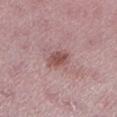Q: Was this lesion biopsied?
A: catalogued during a skin exam; not biopsied
Q: What lighting was used for the tile?
A: white-light
Q: What are the patient's age and sex?
A: female, about 45 years old
Q: What kind of image is this?
A: ~15 mm crop, total-body skin-cancer survey
Q: What is the lesion's diameter?
A: ≈3 mm
Q: What is the anatomic site?
A: the leg